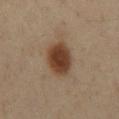body site = the abdomen
lesion size = about 4.5 mm
image-analysis metrics = a footprint of about 13 mm² and two-axis asymmetry of about 0.15
image source = ~15 mm tile from a whole-body skin photo
patient = male, roughly 60 years of age
lighting = cross-polarized illumination